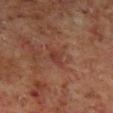This lesion was catalogued during total-body skin photography and was not selected for biopsy. The total-body-photography lesion software estimated a mean CIELAB color near L≈35 a*≈23 b*≈26 and about 6 CIELAB-L* units darker than the surrounding skin. The analysis additionally found a border-irregularity index near 3/10, internal color variation of about 1.5 on a 0–10 scale, and radial color variation of about 0.5. The lesion is on the right lower leg. A male subject, aged 58–62. Measured at roughly 2.5 mm in maximum diameter. A close-up tile cropped from a whole-body skin photograph, about 15 mm across.